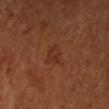Q: Was a biopsy performed?
A: catalogued during a skin exam; not biopsied
Q: How was the tile lit?
A: cross-polarized illumination
Q: What is the lesion's diameter?
A: about 3 mm
Q: Lesion location?
A: the upper back
Q: Patient demographics?
A: male, aged approximately 60
Q: How was this image acquired?
A: ~15 mm crop, total-body skin-cancer survey